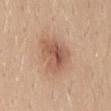Part of a total-body skin-imaging series; this lesion was reviewed on a skin check and was not flagged for biopsy.
Automated image analysis of the tile measured about 11 CIELAB-L* units darker than the surrounding skin and a normalized lesion–skin contrast near 7. The analysis additionally found lesion-presence confidence of about 100/100.
The lesion is located on the mid back.
About 4.5 mm across.
A 15 mm crop from a total-body photograph taken for skin-cancer surveillance.
A female patient, aged around 45.
Captured under white-light illumination.